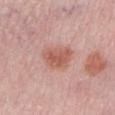Recorded during total-body skin imaging; not selected for excision or biopsy.
The lesion-visualizer software estimated an eccentricity of roughly 0.7 and two-axis asymmetry of about 0.3. The analysis additionally found a mean CIELAB color near L≈57 a*≈24 b*≈28, roughly 10 lightness units darker than nearby skin, and a normalized border contrast of about 7.5.
This is a white-light tile.
This image is a 15 mm lesion crop taken from a total-body photograph.
The lesion is located on the left lower leg.
The subject is a female aged around 45.
The recorded lesion diameter is about 4 mm.